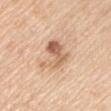follow-up — imaged on a skin check; not biopsied | lesion diameter — ~4.5 mm (longest diameter) | site — the right upper arm | lighting — white-light | image — ~15 mm tile from a whole-body skin photo | subject — male, aged approximately 60.